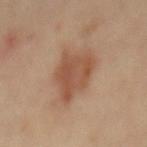Recorded during total-body skin imaging; not selected for excision or biopsy.
Approximately 6 mm at its widest.
A female patient about 45 years old.
Automated image analysis of the tile measured a footprint of about 17 mm², a shape eccentricity near 0.7, and a shape-asymmetry score of about 0.3 (0 = symmetric). It also reported a detector confidence of about 100 out of 100 that the crop contains a lesion.
The tile uses cross-polarized illumination.
This image is a 15 mm lesion crop taken from a total-body photograph.
From the mid back.